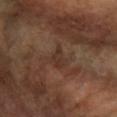Recorded during total-body skin imaging; not selected for excision or biopsy. Measured at roughly 3 mm in maximum diameter. A female subject approximately 60 years of age. Automated image analysis of the tile measured an outline eccentricity of about 0.85 (0 = round, 1 = elongated) and two-axis asymmetry of about 0.45. It also reported a border-irregularity index near 4.5/10 and a color-variation rating of about 2/10. From the right forearm. This image is a 15 mm lesion crop taken from a total-body photograph. Captured under cross-polarized illumination.